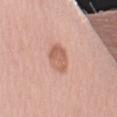Assessment: The lesion was tiled from a total-body skin photograph and was not biopsied. Context: A 15 mm crop from a total-body photograph taken for skin-cancer surveillance. The patient is a female aged 58–62. Located on the left upper arm. Longest diameter approximately 3 mm.